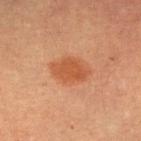The lesion was photographed on a routine skin check and not biopsied; there is no pathology result.
Cropped from a total-body skin-imaging series; the visible field is about 15 mm.
From the right upper arm.
The tile uses cross-polarized illumination.
Longest diameter approximately 4 mm.
The subject is a female about 60 years old.